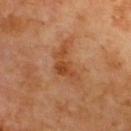workup — catalogued during a skin exam; not biopsied
subject — male, aged around 60
tile lighting — cross-polarized illumination
lesion diameter — ≈5 mm
automated lesion analysis — a lesion area of about 8.5 mm², an outline eccentricity of about 0.85 (0 = round, 1 = elongated), and two-axis asymmetry of about 0.55; an average lesion color of about L≈46 a*≈25 b*≈36 (CIELAB), roughly 9 lightness units darker than nearby skin, and a normalized border contrast of about 7
location — the upper back
acquisition — ~15 mm tile from a whole-body skin photo